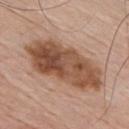The lesion was photographed on a routine skin check and not biopsied; there is no pathology result.